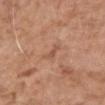Captured during whole-body skin photography for melanoma surveillance; the lesion was not biopsied. The subject is a female aged 83 or older. A 15 mm close-up extracted from a 3D total-body photography capture. The lesion is located on the right upper arm. An algorithmic analysis of the crop reported a footprint of about 2.5 mm² and a shape eccentricity near 0.95. And it measured border irregularity of about 4.5 on a 0–10 scale, a color-variation rating of about 0/10, and a peripheral color-asymmetry measure near 0. Approximately 2.5 mm at its widest. The tile uses white-light illumination.